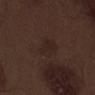Part of a total-body skin-imaging series; this lesion was reviewed on a skin check and was not flagged for biopsy. A male patient, approximately 70 years of age. The lesion is located on the leg. A 15 mm crop from a total-body photograph taken for skin-cancer surveillance.